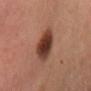Imaged during a routine full-body skin examination; the lesion was not biopsied and no histopathology is available.
This is a cross-polarized tile.
The subject is a female approximately 40 years of age.
Approximately 4 mm at its widest.
From the front of the torso.
A region of skin cropped from a whole-body photographic capture, roughly 15 mm wide.